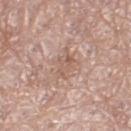No biopsy was performed on this lesion — it was imaged during a full skin examination and was not determined to be concerning.